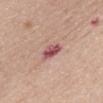Case summary:
– biopsy status · no biopsy performed (imaged during a skin exam)
– automated lesion analysis · internal color variation of about 6 on a 0–10 scale; an automated nevus-likeness rating near 0 out of 100
– illumination · white-light
– anatomic site · the chest
– image source · ~15 mm tile from a whole-body skin photo
– lesion diameter · ~3 mm (longest diameter)
– subject · female, in their mid-60s